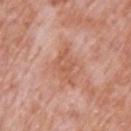Impression:
The lesion was tiled from a total-body skin photograph and was not biopsied.
Background:
The lesion is located on the back. A male patient aged 68–72. The lesion-visualizer software estimated a border-irregularity rating of about 6/10, internal color variation of about 2.5 on a 0–10 scale, and radial color variation of about 1. A close-up tile cropped from a whole-body skin photograph, about 15 mm across. Longest diameter approximately 4 mm. The tile uses white-light illumination.